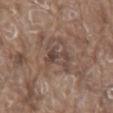Case summary:
– biopsy status: catalogued during a skin exam; not biopsied
– lesion size: ~3.5 mm (longest diameter)
– tile lighting: white-light
– body site: the back
– imaging modality: total-body-photography crop, ~15 mm field of view
– subject: male, approximately 80 years of age
– automated lesion analysis: an average lesion color of about L≈42 a*≈16 b*≈22 (CIELAB) and a lesion–skin lightness drop of about 8; a border-irregularity index near 6.5/10, a within-lesion color-variation index near 1.5/10, and radial color variation of about 0.5